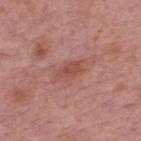Captured during whole-body skin photography for melanoma surveillance; the lesion was not biopsied. The lesion is on the upper back. Imaged with white-light lighting. A region of skin cropped from a whole-body photographic capture, roughly 15 mm wide. A male subject, approximately 75 years of age. An algorithmic analysis of the crop reported an outline eccentricity of about 0.9 (0 = round, 1 = elongated). The analysis additionally found a border-irregularity index near 3/10, a within-lesion color-variation index near 1.5/10, and a peripheral color-asymmetry measure near 0.5. The recorded lesion diameter is about 3.5 mm.